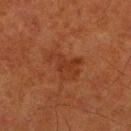automated metrics: a footprint of about 8.5 mm², an outline eccentricity of about 0.75 (0 = round, 1 = elongated), and a shape-asymmetry score of about 0.5 (0 = symmetric); border irregularity of about 5.5 on a 0–10 scale, internal color variation of about 2.5 on a 0–10 scale, and peripheral color asymmetry of about 1 | site: the right lower leg | tile lighting: cross-polarized | lesion size: ≈4.5 mm | patient: male, aged approximately 80 | acquisition: ~15 mm tile from a whole-body skin photo.